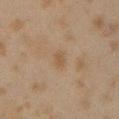| key | value |
|---|---|
| workup | total-body-photography surveillance lesion; no biopsy |